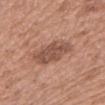Clinical impression:
Recorded during total-body skin imaging; not selected for excision or biopsy.
Acquisition and patient details:
A 15 mm close-up extracted from a 3D total-body photography capture. The recorded lesion diameter is about 5 mm. On the arm. The tile uses white-light illumination. A female patient approximately 60 years of age.